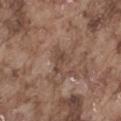Impression:
The lesion was tiled from a total-body skin photograph and was not biopsied.
Background:
The lesion is located on the abdomen. Approximately 3 mm at its widest. A 15 mm close-up tile from a total-body photography series done for melanoma screening. Captured under white-light illumination. The subject is a male aged around 75.